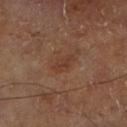No biopsy was performed on this lesion — it was imaged during a full skin examination and was not determined to be concerning. Captured under cross-polarized illumination. A male subject, aged around 70. Cropped from a total-body skin-imaging series; the visible field is about 15 mm. The lesion is on the right lower leg. About 3 mm across. An algorithmic analysis of the crop reported a footprint of about 3 mm², a shape eccentricity near 0.9, and two-axis asymmetry of about 0.3. And it measured internal color variation of about 0.5 on a 0–10 scale and peripheral color asymmetry of about 0. The analysis additionally found an automated nevus-likeness rating near 5 out of 100 and lesion-presence confidence of about 100/100.